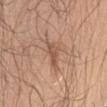Impression: Part of a total-body skin-imaging series; this lesion was reviewed on a skin check and was not flagged for biopsy. Clinical summary: A close-up tile cropped from a whole-body skin photograph, about 15 mm across. Captured under white-light illumination. A male subject aged around 45. The recorded lesion diameter is about 3.5 mm. From the right upper arm.